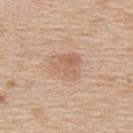Clinical impression: This lesion was catalogued during total-body skin photography and was not selected for biopsy. Background: A male subject aged 58–62. The tile uses white-light illumination. A close-up tile cropped from a whole-body skin photograph, about 15 mm across. An algorithmic analysis of the crop reported a lesion area of about 10 mm² and a symmetry-axis asymmetry near 0.25. The software also gave a mean CIELAB color near L≈62 a*≈18 b*≈31 and about 8 CIELAB-L* units darker than the surrounding skin. And it measured border irregularity of about 2.5 on a 0–10 scale, a within-lesion color-variation index near 4/10, and peripheral color asymmetry of about 1.5. It also reported a nevus-likeness score of about 40/100. The lesion is located on the back. Approximately 4 mm at its widest.